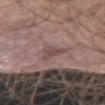The lesion was tiled from a total-body skin photograph and was not biopsied. The subject is a male aged 43–47. A region of skin cropped from a whole-body photographic capture, roughly 15 mm wide. Captured under white-light illumination. On the right upper arm. Approximately 3.5 mm at its widest.